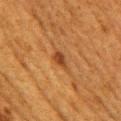{"patient": {"sex": "female", "age_approx": 50}, "site": "right upper arm", "lighting": "cross-polarized", "automated_metrics": {"area_mm2_approx": 3.5, "eccentricity": 0.8, "shape_asymmetry": 0.3, "cielab_L": 38, "cielab_a": 22, "cielab_b": 35, "border_irregularity_0_10": 3.0, "nevus_likeness_0_100": 80, "lesion_detection_confidence_0_100": 100}, "image": {"source": "total-body photography crop", "field_of_view_mm": 15}}